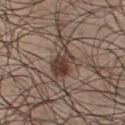Imaged during a routine full-body skin examination; the lesion was not biopsied and no histopathology is available.
This is a white-light tile.
A male subject, aged approximately 25.
Located on the chest.
The lesion's longest dimension is about 6.5 mm.
Cropped from a total-body skin-imaging series; the visible field is about 15 mm.